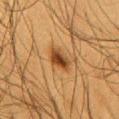Part of a total-body skin-imaging series; this lesion was reviewed on a skin check and was not flagged for biopsy.
Measured at roughly 4 mm in maximum diameter.
A roughly 15 mm field-of-view crop from a total-body skin photograph.
Located on the chest.
A male patient, aged 58 to 62.
Imaged with cross-polarized lighting.